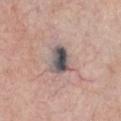A close-up tile cropped from a whole-body skin photograph, about 15 mm across.
Automated image analysis of the tile measured a footprint of about 6.5 mm², an outline eccentricity of about 0.8 (0 = round, 1 = elongated), and a shape-asymmetry score of about 0.2 (0 = symmetric). It also reported a lesion–skin lightness drop of about 18 and a normalized border contrast of about 14. It also reported a nevus-likeness score of about 85/100 and lesion-presence confidence of about 85/100.
A male subject aged 58 to 62.
From the chest.
The recorded lesion diameter is about 3.5 mm.
This is a white-light tile.